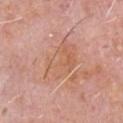Part of a total-body skin-imaging series; this lesion was reviewed on a skin check and was not flagged for biopsy. Imaged with white-light lighting. Measured at roughly 6.5 mm in maximum diameter. A 15 mm close-up extracted from a 3D total-body photography capture. The subject is a male approximately 60 years of age. Located on the chest.